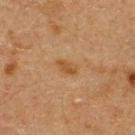This lesion was catalogued during total-body skin photography and was not selected for biopsy.
The total-body-photography lesion software estimated a lesion area of about 3.5 mm², an eccentricity of roughly 0.8, and two-axis asymmetry of about 0.25. It also reported a border-irregularity index near 2.5/10, a within-lesion color-variation index near 1.5/10, and peripheral color asymmetry of about 0.5.
A close-up tile cropped from a whole-body skin photograph, about 15 mm across.
A male patient, approximately 60 years of age.
The lesion's longest dimension is about 2.5 mm.